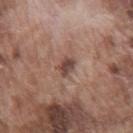Clinical impression:
The lesion was tiled from a total-body skin photograph and was not biopsied.
Background:
The total-body-photography lesion software estimated a footprint of about 3.5 mm², an outline eccentricity of about 0.65 (0 = round, 1 = elongated), and a shape-asymmetry score of about 0.3 (0 = symmetric). And it measured an average lesion color of about L≈45 a*≈19 b*≈23 (CIELAB), about 11 CIELAB-L* units darker than the surrounding skin, and a lesion-to-skin contrast of about 9 (normalized; higher = more distinct). And it measured a lesion-detection confidence of about 100/100. The lesion is located on the chest. A male patient about 75 years old. Captured under white-light illumination. Cropped from a whole-body photographic skin survey; the tile spans about 15 mm. Measured at roughly 2.5 mm in maximum diameter.